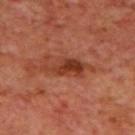This lesion was catalogued during total-body skin photography and was not selected for biopsy.
From the mid back.
Measured at roughly 6 mm in maximum diameter.
A male patient roughly 70 years of age.
The tile uses cross-polarized illumination.
A roughly 15 mm field-of-view crop from a total-body skin photograph.